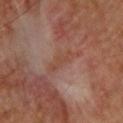<record>
  <biopsy_status>not biopsied; imaged during a skin examination</biopsy_status>
  <image>
    <source>total-body photography crop</source>
    <field_of_view_mm>15</field_of_view_mm>
  </image>
  <lesion_size>
    <long_diameter_mm_approx>3.5</long_diameter_mm_approx>
  </lesion_size>
  <site>back</site>
  <lighting>cross-polarized</lighting>
  <patient>
    <sex>male</sex>
    <age_approx>60</age_approx>
  </patient>
</record>